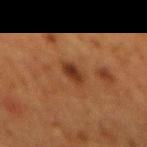{"biopsy_status": "not biopsied; imaged during a skin examination", "image": {"source": "total-body photography crop", "field_of_view_mm": 15}, "site": "mid back", "patient": {"sex": "female", "age_approx": 50}}